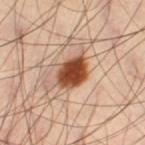Findings:
– workup · total-body-photography surveillance lesion; no biopsy
– body site · the left thigh
– illumination · cross-polarized
– diameter · ~4 mm (longest diameter)
– subject · male, aged approximately 40
– automated metrics · an outline eccentricity of about 0.65 (0 = round, 1 = elongated) and a shape-asymmetry score of about 0.15 (0 = symmetric); a classifier nevus-likeness of about 100/100 and a detector confidence of about 100 out of 100 that the crop contains a lesion
– acquisition · ~15 mm tile from a whole-body skin photo